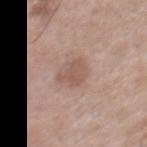Impression:
Part of a total-body skin-imaging series; this lesion was reviewed on a skin check and was not flagged for biopsy.
Clinical summary:
A male subject, aged around 70. On the chest. An algorithmic analysis of the crop reported an area of roughly 6 mm², a shape eccentricity near 0.6, and a symmetry-axis asymmetry near 0.3. And it measured an average lesion color of about L≈55 a*≈19 b*≈25 (CIELAB) and roughly 9 lightness units darker than nearby skin. And it measured internal color variation of about 1.5 on a 0–10 scale and a peripheral color-asymmetry measure near 0.5. A 15 mm crop from a total-body photograph taken for skin-cancer surveillance. The lesion's longest dimension is about 3 mm. The tile uses white-light illumination.